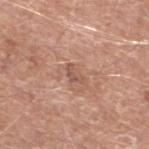Captured during whole-body skin photography for melanoma surveillance; the lesion was not biopsied. From the left lower leg. Cropped from a total-body skin-imaging series; the visible field is about 15 mm. About 2.5 mm across. A male subject, about 80 years old.